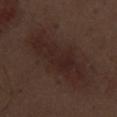This lesion was catalogued during total-body skin photography and was not selected for biopsy.
Located on the left thigh.
Automated image analysis of the tile measured an average lesion color of about L≈23 a*≈16 b*≈18 (CIELAB) and a normalized lesion–skin contrast near 7. It also reported border irregularity of about 4.5 on a 0–10 scale, a color-variation rating of about 2.5/10, and peripheral color asymmetry of about 1.
This is a white-light tile.
The patient is a male roughly 70 years of age.
A 15 mm close-up extracted from a 3D total-body photography capture.